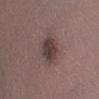{
  "biopsy_status": "not biopsied; imaged during a skin examination",
  "lesion_size": {
    "long_diameter_mm_approx": 3.5
  },
  "automated_metrics": {
    "area_mm2_approx": 8.0,
    "eccentricity": 0.5,
    "shape_asymmetry": 0.1,
    "cielab_L": 38,
    "cielab_a": 16,
    "cielab_b": 17,
    "vs_skin_darker_L": 11.0,
    "vs_skin_contrast_norm": 9.0,
    "border_irregularity_0_10": 1.5,
    "peripheral_color_asymmetry": 1.5,
    "nevus_likeness_0_100": 45,
    "lesion_detection_confidence_0_100": 100
  },
  "patient": {
    "sex": "male",
    "age_approx": 40
  },
  "site": "leg",
  "image": {
    "source": "total-body photography crop",
    "field_of_view_mm": 15
  },
  "lighting": "white-light"
}